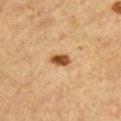<record>
  <patient>
    <sex>male</sex>
    <age_approx>65</age_approx>
  </patient>
  <automated_metrics>
    <area_mm2_approx>3.5</area_mm2_approx>
    <eccentricity>0.8</eccentricity>
    <shape_asymmetry>0.25</shape_asymmetry>
    <cielab_L>46</cielab_L>
    <cielab_a>21</cielab_a>
    <cielab_b>37</cielab_b>
    <vs_skin_darker_L>16.0</vs_skin_darker_L>
    <vs_skin_contrast_norm>11.5</vs_skin_contrast_norm>
    <lesion_detection_confidence_0_100>100</lesion_detection_confidence_0_100>
  </automated_metrics>
  <lesion_size>
    <long_diameter_mm_approx>2.5</long_diameter_mm_approx>
  </lesion_size>
  <image>
    <source>total-body photography crop</source>
    <field_of_view_mm>15</field_of_view_mm>
  </image>
  <site>front of the torso</site>
</record>